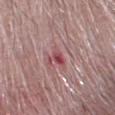imaging modality = ~15 mm tile from a whole-body skin photo
subject = male, aged 68–72
location = the head or neck
illumination = white-light illumination
lesion size = ~2.5 mm (longest diameter)